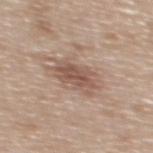No biopsy was performed on this lesion — it was imaged during a full skin examination and was not determined to be concerning.
On the upper back.
A male subject aged around 70.
A roughly 15 mm field-of-view crop from a total-body skin photograph.